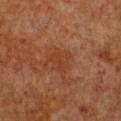location = the chest; patient = female, about 55 years old; illumination = cross-polarized illumination; image = ~15 mm tile from a whole-body skin photo.